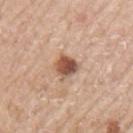<record>
<biopsy_status>not biopsied; imaged during a skin examination</biopsy_status>
<image>
  <source>total-body photography crop</source>
  <field_of_view_mm>15</field_of_view_mm>
</image>
<lighting>white-light</lighting>
<patient>
  <sex>male</sex>
  <age_approx>65</age_approx>
</patient>
<site>left upper arm</site>
</record>